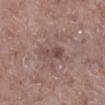  biopsy_status: not biopsied; imaged during a skin examination
  image:
    source: total-body photography crop
    field_of_view_mm: 15
  lighting: white-light
  automated_metrics:
    area_mm2_approx: 5.5
    eccentricity: 0.9
    cielab_L: 47
    cielab_a: 18
    cielab_b: 21
    vs_skin_darker_L: 8.0
    vs_skin_contrast_norm: 6.0
    border_irregularity_0_10: 3.5
    color_variation_0_10: 4.0
    peripheral_color_asymmetry: 1.5
  patient:
    sex: male
    age_approx: 65
  site: right lower leg
  lesion_size:
    long_diameter_mm_approx: 4.0